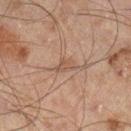| feature | finding |
|---|---|
| biopsy status | imaged on a skin check; not biopsied |
| tile lighting | cross-polarized |
| diameter | ≈2.5 mm |
| patient | male, aged 43 to 47 |
| TBP lesion metrics | a shape eccentricity near 0.85 and a shape-asymmetry score of about 0.4 (0 = symmetric); a classifier nevus-likeness of about 0/100 and lesion-presence confidence of about 65/100 |
| image | ~15 mm crop, total-body skin-cancer survey |
| location | the leg |